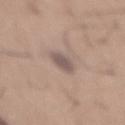Clinical impression: No biopsy was performed on this lesion — it was imaged during a full skin examination and was not determined to be concerning. Background: Automated image analysis of the tile measured an area of roughly 5 mm². The recorded lesion diameter is about 3.5 mm. The lesion is on the mid back. A close-up tile cropped from a whole-body skin photograph, about 15 mm across. A male subject about 65 years old.